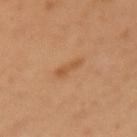Clinical impression:
Captured during whole-body skin photography for melanoma surveillance; the lesion was not biopsied.
Context:
On the arm. A male subject, about 55 years old. A lesion tile, about 15 mm wide, cut from a 3D total-body photograph.